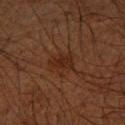biopsy_status: not biopsied; imaged during a skin examination
patient:
  sex: male
  age_approx: 65
lighting: cross-polarized
image:
  source: total-body photography crop
  field_of_view_mm: 15
site: right forearm
automated_metrics:
  area_mm2_approx: 6.0
  eccentricity: 0.7